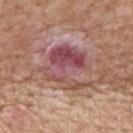Impression:
Part of a total-body skin-imaging series; this lesion was reviewed on a skin check and was not flagged for biopsy.
Clinical summary:
Cropped from a total-body skin-imaging series; the visible field is about 15 mm. A male subject, aged 58–62. The recorded lesion diameter is about 6.5 mm. On the mid back.